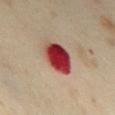Q: Was this lesion biopsied?
A: total-body-photography surveillance lesion; no biopsy